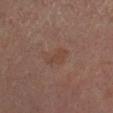Findings:
– follow-up: catalogued during a skin exam; not biopsied
– imaging modality: ~15 mm crop, total-body skin-cancer survey
– diameter: ≈3.5 mm
– site: the right lower leg
– subject: male, in their mid- to late 60s